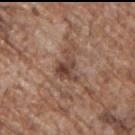{"biopsy_status": "not biopsied; imaged during a skin examination", "lighting": "white-light", "site": "left upper arm", "patient": {"sex": "male", "age_approx": 70}, "image": {"source": "total-body photography crop", "field_of_view_mm": 15}, "automated_metrics": {"eccentricity": 0.7, "cielab_L": 44, "cielab_a": 18, "cielab_b": 25, "vs_skin_darker_L": 11.0}}